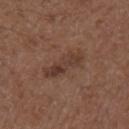biopsy_status: not biopsied; imaged during a skin examination
patient:
  sex: male
  age_approx: 50
image:
  source: total-body photography crop
  field_of_view_mm: 15
site: right upper arm